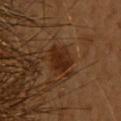Captured during whole-body skin photography for melanoma surveillance; the lesion was not biopsied. A region of skin cropped from a whole-body photographic capture, roughly 15 mm wide. Measured at roughly 4.5 mm in maximum diameter. Imaged with cross-polarized lighting. From the upper back. The subject is a female roughly 55 years of age.